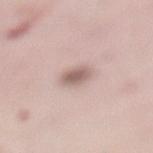Imaged during a routine full-body skin examination; the lesion was not biopsied and no histopathology is available. A female subject approximately 50 years of age. Measured at roughly 2.5 mm in maximum diameter. Automated tile analysis of the lesion measured an average lesion color of about L≈61 a*≈17 b*≈22 (CIELAB) and a lesion–skin lightness drop of about 12. The software also gave a border-irregularity rating of about 1/10, internal color variation of about 2 on a 0–10 scale, and a peripheral color-asymmetry measure near 0.5. The software also gave a nevus-likeness score of about 90/100 and a lesion-detection confidence of about 100/100. The tile uses white-light illumination. The lesion is located on the left lower leg. Cropped from a total-body skin-imaging series; the visible field is about 15 mm.